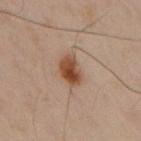Imaged with cross-polarized lighting.
Cropped from a whole-body photographic skin survey; the tile spans about 15 mm.
From the left arm.
The patient is a male aged approximately 50.